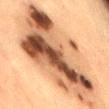Findings:
• workup · no biopsy performed (imaged during a skin exam)
• subject · female, aged 53 to 57
• tile lighting · cross-polarized
• lesion size · ≈13.5 mm
• image · 15 mm crop, total-body photography
• site · the lower back